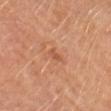follow-up: catalogued during a skin exam; not biopsied
automated lesion analysis: about 7 CIELAB-L* units darker than the surrounding skin and a lesion-to-skin contrast of about 5.5 (normalized; higher = more distinct); lesion-presence confidence of about 100/100
image: ~15 mm crop, total-body skin-cancer survey
body site: the upper back
subject: female, aged approximately 50
diameter: about 2 mm
lighting: cross-polarized